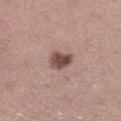The lesion was photographed on a routine skin check and not biopsied; there is no pathology result. The tile uses white-light illumination. An algorithmic analysis of the crop reported border irregularity of about 2.5 on a 0–10 scale, internal color variation of about 4.5 on a 0–10 scale, and peripheral color asymmetry of about 1.5. Longest diameter approximately 3 mm. The lesion is located on the left lower leg. Cropped from a total-body skin-imaging series; the visible field is about 15 mm. The subject is a female roughly 35 years of age.